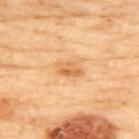Imaged during a routine full-body skin examination; the lesion was not biopsied and no histopathology is available. The patient is a female in their 60s. Cropped from a whole-body photographic skin survey; the tile spans about 15 mm. From the upper back.